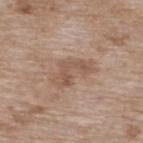A 15 mm close-up extracted from a 3D total-body photography capture. The lesion is located on the back. A female patient aged approximately 75. The recorded lesion diameter is about 5 mm. This is a white-light tile.